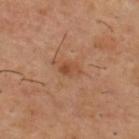Notes:
- follow-up · no biopsy performed (imaged during a skin exam)
- image source · 15 mm crop, total-body photography
- lighting · cross-polarized
- location · the upper back
- size · ~2.5 mm (longest diameter)
- patient · male, about 55 years old